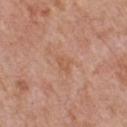This lesion was catalogued during total-body skin photography and was not selected for biopsy.
The lesion is on the chest.
A 15 mm close-up extracted from a 3D total-body photography capture.
A male subject, roughly 60 years of age.